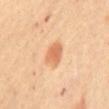Assessment: Part of a total-body skin-imaging series; this lesion was reviewed on a skin check and was not flagged for biopsy. Clinical summary: On the chest. A female subject, approximately 65 years of age. Longest diameter approximately 3.5 mm. Cropped from a total-body skin-imaging series; the visible field is about 15 mm.